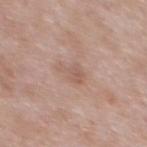| key | value |
|---|---|
| workup | total-body-photography surveillance lesion; no biopsy |
| site | the chest |
| subject | male, approximately 55 years of age |
| image | ~15 mm tile from a whole-body skin photo |
| automated metrics | a lesion area of about 4.5 mm²; a lesion color around L≈57 a*≈18 b*≈26 in CIELAB, roughly 7 lightness units darker than nearby skin, and a lesion-to-skin contrast of about 5 (normalized; higher = more distinct); border irregularity of about 4.5 on a 0–10 scale, a color-variation rating of about 2/10, and radial color variation of about 0.5 |
| size | ~2.5 mm (longest diameter) |